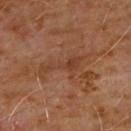Captured during whole-body skin photography for melanoma surveillance; the lesion was not biopsied. Imaged with cross-polarized lighting. An algorithmic analysis of the crop reported a footprint of about 5.5 mm² and an outline eccentricity of about 0.95 (0 = round, 1 = elongated). The analysis additionally found a nevus-likeness score of about 0/100 and a detector confidence of about 100 out of 100 that the crop contains a lesion. Cropped from a total-body skin-imaging series; the visible field is about 15 mm. From the front of the torso. The subject is a male aged approximately 60.